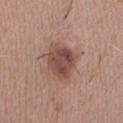This lesion was catalogued during total-body skin photography and was not selected for biopsy.
This image is a 15 mm lesion crop taken from a total-body photograph.
Captured under white-light illumination.
Approximately 5 mm at its widest.
The subject is a male roughly 50 years of age.
An algorithmic analysis of the crop reported a footprint of about 14 mm², a shape eccentricity near 0.65, and a symmetry-axis asymmetry near 0.15.
From the right upper arm.